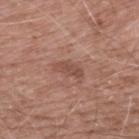Q: Was a biopsy performed?
A: imaged on a skin check; not biopsied
Q: How large is the lesion?
A: ~3.5 mm (longest diameter)
Q: Illumination type?
A: white-light
Q: Patient demographics?
A: male, roughly 60 years of age
Q: What is the anatomic site?
A: the upper back
Q: What is the imaging modality?
A: 15 mm crop, total-body photography